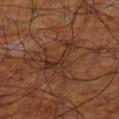Clinical impression: Part of a total-body skin-imaging series; this lesion was reviewed on a skin check and was not flagged for biopsy. Background: The recorded lesion diameter is about 5.5 mm. The patient is a male aged approximately 60. A lesion tile, about 15 mm wide, cut from a 3D total-body photograph. The lesion is located on the leg. Automated tile analysis of the lesion measured an area of roughly 9.5 mm², an eccentricity of roughly 0.85, and two-axis asymmetry of about 0.75. The software also gave a mean CIELAB color near L≈31 a*≈20 b*≈28, a lesion–skin lightness drop of about 6, and a normalized border contrast of about 6.5. Imaged with cross-polarized lighting.